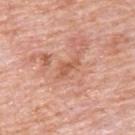{"biopsy_status": "not biopsied; imaged during a skin examination", "site": "upper back", "automated_metrics": {"area_mm2_approx": 4.0, "eccentricity": 0.9, "shape_asymmetry": 0.5}, "image": {"source": "total-body photography crop", "field_of_view_mm": 15}, "lesion_size": {"long_diameter_mm_approx": 3.5}, "lighting": "white-light", "patient": {"sex": "male", "age_approx": 80}}